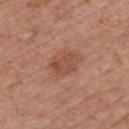<record>
<biopsy_status>not biopsied; imaged during a skin examination</biopsy_status>
<patient>
  <sex>female</sex>
  <age_approx>40</age_approx>
</patient>
<lighting>white-light</lighting>
<lesion_size>
  <long_diameter_mm_approx>3.5</long_diameter_mm_approx>
</lesion_size>
<image>
  <source>total-body photography crop</source>
  <field_of_view_mm>15</field_of_view_mm>
</image>
<site>upper back</site>
</record>